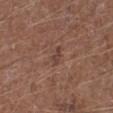Impression:
The lesion was tiled from a total-body skin photograph and was not biopsied.
Context:
A male patient about 75 years old. A lesion tile, about 15 mm wide, cut from a 3D total-body photograph. An algorithmic analysis of the crop reported a shape eccentricity near 0.9 and a shape-asymmetry score of about 0.4 (0 = symmetric). And it measured a border-irregularity rating of about 4.5/10. It also reported an automated nevus-likeness rating near 0 out of 100. Measured at roughly 2.5 mm in maximum diameter. The lesion is on the left lower leg.